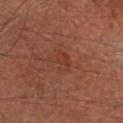Impression: Part of a total-body skin-imaging series; this lesion was reviewed on a skin check and was not flagged for biopsy. Image and clinical context: The patient is a male in their 60s. A close-up tile cropped from a whole-body skin photograph, about 15 mm across. The recorded lesion diameter is about 2.5 mm. An algorithmic analysis of the crop reported a border-irregularity rating of about 6/10, a within-lesion color-variation index near 1.5/10, and peripheral color asymmetry of about 0.5. It also reported a classifier nevus-likeness of about 0/100 and lesion-presence confidence of about 100/100. Imaged with cross-polarized lighting. Located on the head or neck.